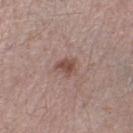biopsy status = no biopsy performed (imaged during a skin exam) | imaging modality = ~15 mm tile from a whole-body skin photo | site = the left lower leg | patient = male, approximately 45 years of age | diameter = ~2.5 mm (longest diameter) | automated metrics = a lesion area of about 4 mm² and a symmetry-axis asymmetry near 0.4; a color-variation rating of about 2/10 and a peripheral color-asymmetry measure near 1; a nevus-likeness score of about 45/100 and a lesion-detection confidence of about 100/100.